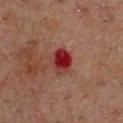No biopsy was performed on this lesion — it was imaged during a full skin examination and was not determined to be concerning.
Cropped from a total-body skin-imaging series; the visible field is about 15 mm.
Located on the chest.
The patient is a male approximately 50 years of age.